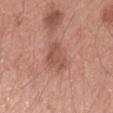The lesion was tiled from a total-body skin photograph and was not biopsied. A roughly 15 mm field-of-view crop from a total-body skin photograph. Longest diameter approximately 3.5 mm. The lesion is on the arm. The tile uses white-light illumination. A female patient, aged approximately 40.